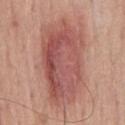| feature | finding |
|---|---|
| workup | imaged on a skin check; not biopsied |
| acquisition | total-body-photography crop, ~15 mm field of view |
| TBP lesion metrics | a lesion area of about 43 mm² and an eccentricity of roughly 0.85; a mean CIELAB color near L≈53 a*≈26 b*≈25 and a normalized border contrast of about 8; radial color variation of about 2.5; a nevus-likeness score of about 45/100 and lesion-presence confidence of about 100/100 |
| patient | male, aged 53–57 |
| body site | the mid back |
| diameter | ~10.5 mm (longest diameter) |